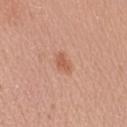Recorded during total-body skin imaging; not selected for excision or biopsy. A roughly 15 mm field-of-view crop from a total-body skin photograph. Imaged with white-light lighting. Located on the left upper arm. A female patient, aged approximately 40.